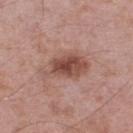Q: What are the patient's age and sex?
A: male, roughly 55 years of age
Q: How large is the lesion?
A: about 6 mm
Q: What kind of image is this?
A: ~15 mm tile from a whole-body skin photo
Q: What lighting was used for the tile?
A: white-light illumination
Q: Where on the body is the lesion?
A: the left lower leg